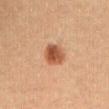- biopsy status · imaged on a skin check; not biopsied
- automated metrics · a footprint of about 6.5 mm², an eccentricity of roughly 0.55, and a shape-asymmetry score of about 0.15 (0 = symmetric); border irregularity of about 1 on a 0–10 scale, internal color variation of about 4.5 on a 0–10 scale, and radial color variation of about 1.5; a nevus-likeness score of about 100/100 and lesion-presence confidence of about 100/100
- site · the front of the torso
- subject · female, aged 18 to 22
- acquisition · total-body-photography crop, ~15 mm field of view
- illumination · cross-polarized illumination
- lesion size · ~3 mm (longest diameter)